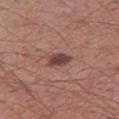follow-up: no biopsy performed (imaged during a skin exam)
patient: male, aged 53 to 57
site: the leg
image: 15 mm crop, total-body photography
lesion diameter: ~3 mm (longest diameter)
lighting: white-light illumination
image-analysis metrics: an area of roughly 4.5 mm², a shape eccentricity near 0.75, and a shape-asymmetry score of about 0.3 (0 = symmetric); a border-irregularity rating of about 3/10, a within-lesion color-variation index near 3/10, and a peripheral color-asymmetry measure near 1